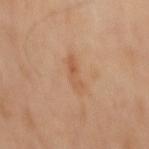• follow-up · no biopsy performed (imaged during a skin exam)
• image · ~15 mm crop, total-body skin-cancer survey
• lesion size · ~4 mm (longest diameter)
• automated lesion analysis · an area of roughly 4 mm² and an outline eccentricity of about 0.95 (0 = round, 1 = elongated); a border-irregularity rating of about 4.5/10 and a color-variation rating of about 2/10; a lesion-detection confidence of about 100/100
• illumination · cross-polarized
• location · the mid back